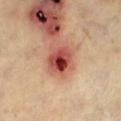follow-up: imaged on a skin check; not biopsied
patient: about 60 years old
diameter: about 3.5 mm
tile lighting: cross-polarized
anatomic site: the leg
TBP lesion metrics: an automated nevus-likeness rating near 0 out of 100 and a detector confidence of about 100 out of 100 that the crop contains a lesion
image: 15 mm crop, total-body photography